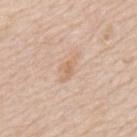No biopsy was performed on this lesion — it was imaged during a full skin examination and was not determined to be concerning.
The lesion is located on the mid back.
A close-up tile cropped from a whole-body skin photograph, about 15 mm across.
A male patient about 80 years old.
This is a white-light tile.
The total-body-photography lesion software estimated a footprint of about 4.5 mm² and two-axis asymmetry of about 0.45. And it measured a border-irregularity rating of about 5/10, a within-lesion color-variation index near 2/10, and radial color variation of about 0.5.